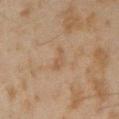Assessment:
Recorded during total-body skin imaging; not selected for excision or biopsy.
Background:
A roughly 15 mm field-of-view crop from a total-body skin photograph. On the arm. A male patient aged 43 to 47. The tile uses cross-polarized illumination.